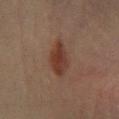Assessment: The lesion was tiled from a total-body skin photograph and was not biopsied. Acquisition and patient details: About 5 mm across. A 15 mm close-up extracted from a 3D total-body photography capture. A male patient in their 60s. Imaged with cross-polarized lighting. On the left lower leg.